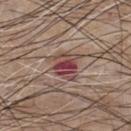biopsy_status: not biopsied; imaged during a skin examination
image:
  source: total-body photography crop
  field_of_view_mm: 15
lighting: white-light
site: chest
lesion_size:
  long_diameter_mm_approx: 4.0
patient:
  sex: male
  age_approx: 65
automated_metrics:
  cielab_L: 45
  cielab_a: 22
  cielab_b: 21
  vs_skin_contrast_norm: 9.0